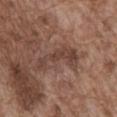biopsy_status: not biopsied; imaged during a skin examination
automated_metrics:
  border_irregularity_0_10: 8.0
  color_variation_0_10: 2.0
  peripheral_color_asymmetry: 0.5
image:
  source: total-body photography crop
  field_of_view_mm: 15
lesion_size:
  long_diameter_mm_approx: 5.5
patient:
  sex: male
  age_approx: 75
lighting: white-light
site: abdomen